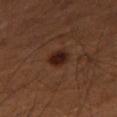workup = total-body-photography surveillance lesion; no biopsy
anatomic site = the leg
subject = male, aged 53–57
image = ~15 mm tile from a whole-body skin photo
tile lighting = cross-polarized illumination
lesion size = ≈3 mm